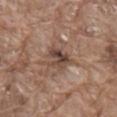image-analysis metrics: a footprint of about 7.5 mm², an eccentricity of roughly 0.45, and a shape-asymmetry score of about 0.25 (0 = symmetric)
illumination: white-light illumination
location: the back
subject: male, about 80 years old
imaging modality: ~15 mm tile from a whole-body skin photo
size: about 3.5 mm